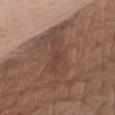Recorded during total-body skin imaging; not selected for excision or biopsy.
The lesion is located on the chest.
This image is a 15 mm lesion crop taken from a total-body photograph.
A female subject aged 73–77.